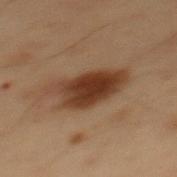Q: Is there a histopathology result?
A: no biopsy performed (imaged during a skin exam)
Q: What kind of image is this?
A: total-body-photography crop, ~15 mm field of view
Q: What are the patient's age and sex?
A: male, in their mid- to late 50s
Q: Lesion location?
A: the mid back
Q: How was the tile lit?
A: cross-polarized